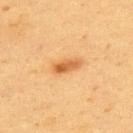This lesion was catalogued during total-body skin photography and was not selected for biopsy. A 15 mm crop from a total-body photograph taken for skin-cancer surveillance. Imaged with cross-polarized lighting. A female patient, aged around 40. On the upper back.